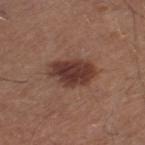{"biopsy_status": "not biopsied; imaged during a skin examination", "site": "left thigh", "image": {"source": "total-body photography crop", "field_of_view_mm": 15}, "lighting": "white-light", "lesion_size": {"long_diameter_mm_approx": 5.0}, "patient": {"sex": "male", "age_approx": 65}, "automated_metrics": {"cielab_L": 36, "cielab_a": 21, "cielab_b": 23, "vs_skin_darker_L": 13.0, "vs_skin_contrast_norm": 10.5}}